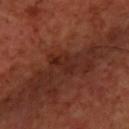This lesion was catalogued during total-body skin photography and was not selected for biopsy.
Approximately 3.5 mm at its widest.
From the upper back.
A male patient aged around 70.
A lesion tile, about 15 mm wide, cut from a 3D total-body photograph.